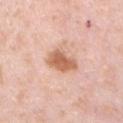Part of a total-body skin-imaging series; this lesion was reviewed on a skin check and was not flagged for biopsy. On the chest. A male subject aged 33 to 37. A close-up tile cropped from a whole-body skin photograph, about 15 mm across.